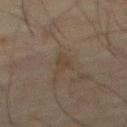follow-up: imaged on a skin check; not biopsied | body site: the abdomen | illumination: cross-polarized | lesion diameter: ≈3.5 mm | image-analysis metrics: peripheral color asymmetry of about 0.5; a detector confidence of about 85 out of 100 that the crop contains a lesion | image source: total-body-photography crop, ~15 mm field of view | subject: male, aged 68 to 72.